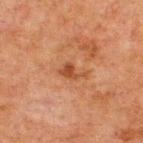follow-up=total-body-photography surveillance lesion; no biopsy
lighting=cross-polarized
size=~3 mm (longest diameter)
subject=male, aged approximately 60
anatomic site=the upper back
image source=total-body-photography crop, ~15 mm field of view